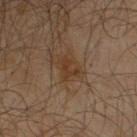The total-body-photography lesion software estimated an eccentricity of roughly 0.7 and two-axis asymmetry of about 0.5. It also reported border irregularity of about 6.5 on a 0–10 scale, a color-variation rating of about 0/10, and peripheral color asymmetry of about 0. The software also gave a classifier nevus-likeness of about 10/100 and a lesion-detection confidence of about 100/100. A 15 mm crop from a total-body photograph taken for skin-cancer surveillance. The recorded lesion diameter is about 3 mm. Captured under cross-polarized illumination. The patient is a male about 65 years old. The lesion is located on the right upper arm.